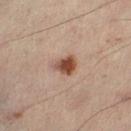Assessment: Imaged during a routine full-body skin examination; the lesion was not biopsied and no histopathology is available. Acquisition and patient details: The subject is a male aged 48–52. Captured under cross-polarized illumination. Measured at roughly 3 mm in maximum diameter. On the left lower leg. The lesion-visualizer software estimated an area of roughly 5 mm², a shape eccentricity near 0.7, and two-axis asymmetry of about 0.3. The software also gave a lesion color around L≈43 a*≈19 b*≈26 in CIELAB. And it measured a border-irregularity rating of about 3/10. The software also gave a classifier nevus-likeness of about 95/100 and a lesion-detection confidence of about 100/100. Cropped from a whole-body photographic skin survey; the tile spans about 15 mm.